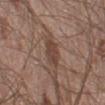| key | value |
|---|---|
| image | total-body-photography crop, ~15 mm field of view |
| lesion diameter | ~3 mm (longest diameter) |
| site | the left lower leg |
| illumination | white-light |
| automated lesion analysis | a lesion area of about 5 mm², an outline eccentricity of about 0.75 (0 = round, 1 = elongated), and a shape-asymmetry score of about 0.3 (0 = symmetric); a nevus-likeness score of about 50/100 and a lesion-detection confidence of about 100/100 |
| patient | male, approximately 20 years of age |